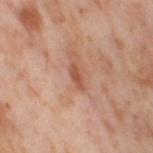notes: catalogued during a skin exam; not biopsied
image: ~15 mm tile from a whole-body skin photo
diameter: about 3 mm
lighting: cross-polarized
patient: female, approximately 55 years of age
location: the right thigh